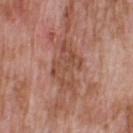Assessment:
The lesion was photographed on a routine skin check and not biopsied; there is no pathology result.
Image and clinical context:
A male subject, in their 60s. This is a white-light tile. A lesion tile, about 15 mm wide, cut from a 3D total-body photograph. Longest diameter approximately 6.5 mm. Located on the back. Automated tile analysis of the lesion measured border irregularity of about 5 on a 0–10 scale, internal color variation of about 4 on a 0–10 scale, and a peripheral color-asymmetry measure near 1.5.